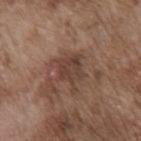Assessment: No biopsy was performed on this lesion — it was imaged during a full skin examination and was not determined to be concerning. Acquisition and patient details: This is a white-light tile. From the chest. Cropped from a total-body skin-imaging series; the visible field is about 15 mm. The patient is a male aged approximately 75.